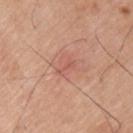– notes: catalogued during a skin exam; not biopsied
– automated metrics: a lesion area of about 2 mm², an outline eccentricity of about 0.95 (0 = round, 1 = elongated), and a shape-asymmetry score of about 0.55 (0 = symmetric); a lesion color around L≈56 a*≈27 b*≈28 in CIELAB and a lesion-to-skin contrast of about 5 (normalized; higher = more distinct); a border-irregularity rating of about 6.5/10, internal color variation of about 0 on a 0–10 scale, and peripheral color asymmetry of about 0
– tile lighting: white-light
– subject: male, aged 73 to 77
– body site: the upper back
– size: about 2.5 mm
– image source: 15 mm crop, total-body photography